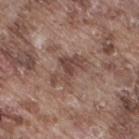Background:
About 5 mm across. This image is a 15 mm lesion crop taken from a total-body photograph. This is a white-light tile. On the right thigh. Automated tile analysis of the lesion measured an area of roughly 8.5 mm², an outline eccentricity of about 0.85 (0 = round, 1 = elongated), and a shape-asymmetry score of about 0.55 (0 = symmetric). The software also gave an average lesion color of about L≈45 a*≈18 b*≈23 (CIELAB), a lesion–skin lightness drop of about 10, and a normalized border contrast of about 7.5. It also reported border irregularity of about 6.5 on a 0–10 scale, a color-variation rating of about 2.5/10, and a peripheral color-asymmetry measure near 0.5. The analysis additionally found a nevus-likeness score of about 0/100. A male patient, roughly 75 years of age.